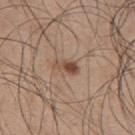The lesion was tiled from a total-body skin photograph and was not biopsied. The tile uses white-light illumination. On the upper back. A male subject, aged approximately 50. A roughly 15 mm field-of-view crop from a total-body skin photograph.